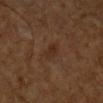{
  "biopsy_status": "not biopsied; imaged during a skin examination",
  "lesion_size": {
    "long_diameter_mm_approx": 2.5
  },
  "site": "arm",
  "patient": {
    "sex": "male",
    "age_approx": 65
  },
  "image": {
    "source": "total-body photography crop",
    "field_of_view_mm": 15
  },
  "automated_metrics": {
    "eccentricity": 0.7,
    "nevus_likeness_0_100": 10,
    "lesion_detection_confidence_0_100": 100
  }
}